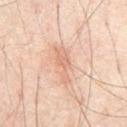biopsy status: imaged on a skin check; not biopsied
subject: male, roughly 65 years of age
anatomic site: the abdomen
TBP lesion metrics: a mean CIELAB color near L≈66 a*≈21 b*≈30, roughly 8 lightness units darker than nearby skin, and a normalized lesion–skin contrast near 5
diameter: ~4.5 mm (longest diameter)
image source: total-body-photography crop, ~15 mm field of view
illumination: cross-polarized illumination